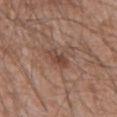{
  "biopsy_status": "not biopsied; imaged during a skin examination",
  "site": "mid back",
  "patient": {
    "sex": "male",
    "age_approx": 60
  },
  "image": {
    "source": "total-body photography crop",
    "field_of_view_mm": 15
  },
  "lesion_size": {
    "long_diameter_mm_approx": 3.0
  },
  "lighting": "white-light"
}